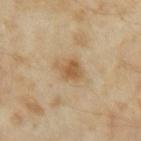Impression: The lesion was tiled from a total-body skin photograph and was not biopsied. Clinical summary: Approximately 3.5 mm at its widest. The tile uses cross-polarized illumination. A close-up tile cropped from a whole-body skin photograph, about 15 mm across. A female subject, aged around 35. On the right upper arm.